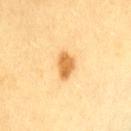The lesion was photographed on a routine skin check and not biopsied; there is no pathology result.
A female subject, aged around 55.
The lesion is on the lower back.
A 15 mm close-up extracted from a 3D total-body photography capture.
Longest diameter approximately 3.5 mm.
The tile uses cross-polarized illumination.